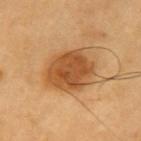The lesion was tiled from a total-body skin photograph and was not biopsied. From the left upper arm. Approximately 5.5 mm at its widest. The tile uses cross-polarized illumination. A male subject roughly 60 years of age. Cropped from a whole-body photographic skin survey; the tile spans about 15 mm.